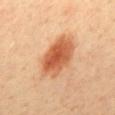diameter: ~5.5 mm (longest diameter) | location: the back | patient: male, in their 40s | image-analysis metrics: a lesion area of about 17 mm², an eccentricity of roughly 0.8, and a shape-asymmetry score of about 0.15 (0 = symmetric); a mean CIELAB color near L≈59 a*≈29 b*≈40 and about 14 CIELAB-L* units darker than the surrounding skin; a nevus-likeness score of about 100/100 and a lesion-detection confidence of about 100/100 | image: total-body-photography crop, ~15 mm field of view | tile lighting: cross-polarized illumination.